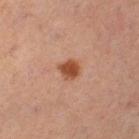Part of a total-body skin-imaging series; this lesion was reviewed on a skin check and was not flagged for biopsy. Imaged with cross-polarized lighting. Cropped from a whole-body photographic skin survey; the tile spans about 15 mm. Longest diameter approximately 2.5 mm. A female patient, aged 53–57. Automated tile analysis of the lesion measured an outline eccentricity of about 0.25 (0 = round, 1 = elongated). The software also gave an automated nevus-likeness rating near 100 out of 100 and a detector confidence of about 100 out of 100 that the crop contains a lesion. From the left lower leg.